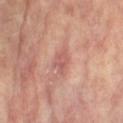Part of a total-body skin-imaging series; this lesion was reviewed on a skin check and was not flagged for biopsy. A close-up tile cropped from a whole-body skin photograph, about 15 mm across. Imaged with cross-polarized lighting. The subject is a female aged approximately 75. From the right lower leg.